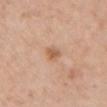Captured during whole-body skin photography for melanoma surveillance; the lesion was not biopsied.
The lesion-visualizer software estimated an area of roughly 3.5 mm². And it measured border irregularity of about 3 on a 0–10 scale and radial color variation of about 0.5. It also reported a classifier nevus-likeness of about 55/100.
A 15 mm close-up extracted from a 3D total-body photography capture.
This is a white-light tile.
Approximately 2.5 mm at its widest.
The subject is a female aged around 65.
The lesion is on the chest.